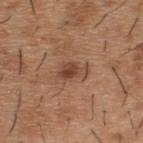{
  "biopsy_status": "not biopsied; imaged during a skin examination",
  "patient": {
    "sex": "male",
    "age_approx": 40
  },
  "lesion_size": {
    "long_diameter_mm_approx": 3.5
  },
  "site": "upper back",
  "image": {
    "source": "total-body photography crop",
    "field_of_view_mm": 15
  }
}